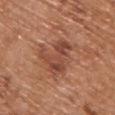Impression: Recorded during total-body skin imaging; not selected for excision or biopsy. Background: Cropped from a whole-body photographic skin survey; the tile spans about 15 mm. The lesion's longest dimension is about 4.5 mm. Automated image analysis of the tile measured a shape-asymmetry score of about 0.5 (0 = symmetric). And it measured a nevus-likeness score of about 5/100 and lesion-presence confidence of about 100/100. The subject is a male aged approximately 70. Captured under white-light illumination. From the upper back.